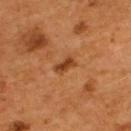Background:
A region of skin cropped from a whole-body photographic capture, roughly 15 mm wide. The lesion's longest dimension is about 2.5 mm. A male subject, aged around 55. An algorithmic analysis of the crop reported an outline eccentricity of about 0.85 (0 = round, 1 = elongated). The software also gave an average lesion color of about L≈39 a*≈25 b*≈37 (CIELAB) and a lesion-to-skin contrast of about 8.5 (normalized; higher = more distinct). On the upper back.